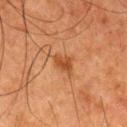{"biopsy_status": "not biopsied; imaged during a skin examination", "site": "left thigh", "lighting": "cross-polarized", "lesion_size": {"long_diameter_mm_approx": 2.5}, "patient": {"sex": "male", "age_approx": 80}, "automated_metrics": {"area_mm2_approx": 4.0, "eccentricity": 0.75, "shape_asymmetry": 0.35, "cielab_L": 38, "cielab_a": 23, "cielab_b": 33, "vs_skin_darker_L": 8.0}, "image": {"source": "total-body photography crop", "field_of_view_mm": 15}}